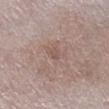The lesion was tiled from a total-body skin photograph and was not biopsied.
A male subject about 80 years old.
A 15 mm close-up extracted from a 3D total-body photography capture.
From the right lower leg.